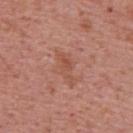| field | value |
|---|---|
| notes | catalogued during a skin exam; not biopsied |
| lighting | white-light |
| acquisition | ~15 mm crop, total-body skin-cancer survey |
| anatomic site | the upper back |
| subject | male, approximately 70 years of age |